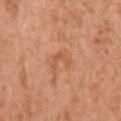Clinical impression: Recorded during total-body skin imaging; not selected for excision or biopsy. Image and clinical context: On the arm. The subject is a female approximately 65 years of age. This image is a 15 mm lesion crop taken from a total-body photograph.